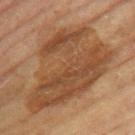Imaged during a routine full-body skin examination; the lesion was not biopsied and no histopathology is available. A 15 mm close-up extracted from a 3D total-body photography capture. Captured under cross-polarized illumination. A female subject, aged 68–72. Located on the right thigh.